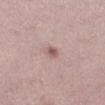Captured during whole-body skin photography for melanoma surveillance; the lesion was not biopsied.
The total-body-photography lesion software estimated a shape eccentricity near 0.6 and a symmetry-axis asymmetry near 0.3. And it measured about 11 CIELAB-L* units darker than the surrounding skin and a lesion-to-skin contrast of about 7 (normalized; higher = more distinct). The analysis additionally found a detector confidence of about 100 out of 100 that the crop contains a lesion.
On the right lower leg.
This is a white-light tile.
Cropped from a total-body skin-imaging series; the visible field is about 15 mm.
A female subject, roughly 20 years of age.
Measured at roughly 2 mm in maximum diameter.